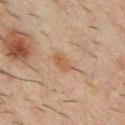* follow-up · total-body-photography surveillance lesion; no biopsy
* image · 15 mm crop, total-body photography
* patient · male, aged around 35
* location · the chest